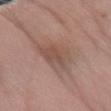Findings:
* acquisition · ~15 mm crop, total-body skin-cancer survey
* site · the right forearm
* patient · female, roughly 60 years of age
* lesion size · about 5 mm